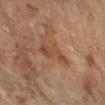Q: Was this lesion biopsied?
A: no biopsy performed (imaged during a skin exam)
Q: What did automated image analysis measure?
A: a footprint of about 6 mm², an outline eccentricity of about 0.9 (0 = round, 1 = elongated), and two-axis asymmetry of about 0.4; a mean CIELAB color near L≈48 a*≈21 b*≈32, about 8 CIELAB-L* units darker than the surrounding skin, and a lesion-to-skin contrast of about 6 (normalized; higher = more distinct); a border-irregularity index near 5/10, a color-variation rating of about 3/10, and a peripheral color-asymmetry measure near 1; a classifier nevus-likeness of about 0/100 and a lesion-detection confidence of about 100/100
Q: Lesion location?
A: the right forearm
Q: What are the patient's age and sex?
A: female, aged approximately 60
Q: How was this image acquired?
A: total-body-photography crop, ~15 mm field of view